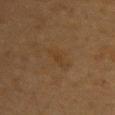On the upper back. A male subject aged approximately 85 or older. A region of skin cropped from a whole-body photographic capture, roughly 15 mm wide. The lesion-visualizer software estimated an area of roughly 2.5 mm², an outline eccentricity of about 0.95 (0 = round, 1 = elongated), and two-axis asymmetry of about 0.45. Longest diameter approximately 3 mm.